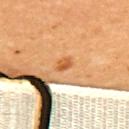Captured during whole-body skin photography for melanoma surveillance; the lesion was not biopsied. A female subject, roughly 30 years of age. About 2 mm across. On the upper back. Captured under cross-polarized illumination. A close-up tile cropped from a whole-body skin photograph, about 15 mm across.